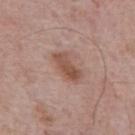Assessment: The lesion was tiled from a total-body skin photograph and was not biopsied. Clinical summary: Captured under white-light illumination. A male patient, roughly 65 years of age. Approximately 4 mm at its widest. A lesion tile, about 15 mm wide, cut from a 3D total-body photograph. From the mid back. The total-body-photography lesion software estimated a lesion color around L≈51 a*≈21 b*≈27 in CIELAB, a lesion–skin lightness drop of about 10, and a normalized border contrast of about 8. The analysis additionally found a border-irregularity index near 2.5/10, a color-variation rating of about 4/10, and a peripheral color-asymmetry measure near 1.5. It also reported an automated nevus-likeness rating near 50 out of 100 and lesion-presence confidence of about 100/100.